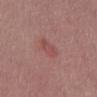Impression:
The lesion was photographed on a routine skin check and not biopsied; there is no pathology result.
Background:
On the mid back. The total-body-photography lesion software estimated border irregularity of about 3 on a 0–10 scale, internal color variation of about 2 on a 0–10 scale, and a peripheral color-asymmetry measure near 0.5. The analysis additionally found an automated nevus-likeness rating near 15 out of 100 and a detector confidence of about 100 out of 100 that the crop contains a lesion. The patient is a male in their mid-50s. A lesion tile, about 15 mm wide, cut from a 3D total-body photograph. Imaged with white-light lighting.